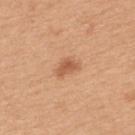lighting: white-light illumination | acquisition: ~15 mm tile from a whole-body skin photo | patient: female, roughly 30 years of age | lesion size: about 3 mm | automated metrics: border irregularity of about 3.5 on a 0–10 scale and a within-lesion color-variation index near 1.5/10; a nevus-likeness score of about 90/100 | anatomic site: the upper back.